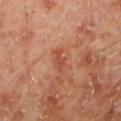Part of a total-body skin-imaging series; this lesion was reviewed on a skin check and was not flagged for biopsy.
A male patient approximately 70 years of age.
Located on the left lower leg.
A 15 mm crop from a total-body photograph taken for skin-cancer surveillance.
Imaged with cross-polarized lighting.